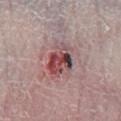The lesion-visualizer software estimated an eccentricity of roughly 0.8 and a symmetry-axis asymmetry near 0.25. And it measured a mean CIELAB color near L≈48 a*≈24 b*≈19 and a lesion–skin lightness drop of about 13. It also reported a classifier nevus-likeness of about 0/100 and a detector confidence of about 100 out of 100 that the crop contains a lesion.
From the left lower leg.
The recorded lesion diameter is about 5.5 mm.
A 15 mm crop from a total-body photograph taken for skin-cancer surveillance.
The patient is a male aged 78 to 82.
Captured under white-light illumination.